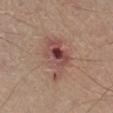Impression:
Recorded during total-body skin imaging; not selected for excision or biopsy.
Background:
A male subject aged 53 to 57. Imaged with white-light lighting. From the leg. A 15 mm crop from a total-body photograph taken for skin-cancer surveillance. Automated tile analysis of the lesion measured a lesion color around L≈48 a*≈22 b*≈22 in CIELAB, roughly 11 lightness units darker than nearby skin, and a lesion-to-skin contrast of about 8.5 (normalized; higher = more distinct). And it measured a classifier nevus-likeness of about 0/100 and lesion-presence confidence of about 100/100. About 5.5 mm across.